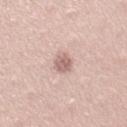The lesion was tiled from a total-body skin photograph and was not biopsied. About 2.5 mm across. A 15 mm close-up tile from a total-body photography series done for melanoma screening. This is a white-light tile. The lesion is located on the right upper arm. Automated image analysis of the tile measured a lesion area of about 4 mm², an eccentricity of roughly 0.65, and a shape-asymmetry score of about 0.2 (0 = symmetric). And it measured a lesion–skin lightness drop of about 12 and a lesion-to-skin contrast of about 7.5 (normalized; higher = more distinct). A female subject aged around 35.